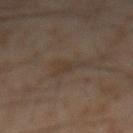{
  "biopsy_status": "not biopsied; imaged during a skin examination",
  "patient": {
    "sex": "male",
    "age_approx": 60
  },
  "lighting": "cross-polarized",
  "lesion_size": {
    "long_diameter_mm_approx": 3.5
  },
  "automated_metrics": {
    "eccentricity": 0.75,
    "shape_asymmetry": 0.55,
    "border_irregularity_0_10": 5.5,
    "peripheral_color_asymmetry": 0.5
  },
  "image": {
    "source": "total-body photography crop",
    "field_of_view_mm": 15
  },
  "site": "abdomen"
}